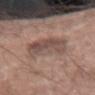Imaged during a routine full-body skin examination; the lesion was not biopsied and no histopathology is available.
Automated image analysis of the tile measured an area of roughly 16 mm², an eccentricity of roughly 0.8, and two-axis asymmetry of about 0.25. And it measured a mean CIELAB color near L≈49 a*≈17 b*≈22 and roughly 10 lightness units darker than nearby skin. And it measured border irregularity of about 3.5 on a 0–10 scale, a color-variation rating of about 5/10, and a peripheral color-asymmetry measure near 2.
Cropped from a whole-body photographic skin survey; the tile spans about 15 mm.
The lesion is on the right forearm.
This is a white-light tile.
The subject is a male aged 48 to 52.
Longest diameter approximately 6 mm.